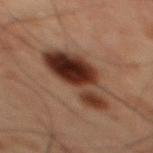{
  "biopsy_status": "not biopsied; imaged during a skin examination",
  "lighting": "cross-polarized",
  "lesion_size": {
    "long_diameter_mm_approx": 7.0
  },
  "site": "mid back",
  "image": {
    "source": "total-body photography crop",
    "field_of_view_mm": 15
  },
  "patient": {
    "sex": "male",
    "age_approx": 60
  }
}